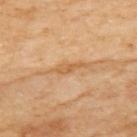workup = no biopsy performed (imaged during a skin exam)
body site = the upper back
patient = female, approximately 60 years of age
imaging modality = ~15 mm crop, total-body skin-cancer survey
lesion diameter = about 3 mm
illumination = cross-polarized